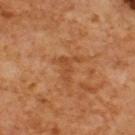The lesion was photographed on a routine skin check and not biopsied; there is no pathology result. Measured at roughly 3.5 mm in maximum diameter. A male patient, aged approximately 60. The lesion is on the upper back. Cropped from a total-body skin-imaging series; the visible field is about 15 mm. An algorithmic analysis of the crop reported an outline eccentricity of about 0.7 (0 = round, 1 = elongated) and a shape-asymmetry score of about 0.6 (0 = symmetric). The software also gave a lesion color around L≈43 a*≈23 b*≈36 in CIELAB, a lesion–skin lightness drop of about 6, and a lesion-to-skin contrast of about 5 (normalized; higher = more distinct). The analysis additionally found a border-irregularity index near 8/10, internal color variation of about 1.5 on a 0–10 scale, and radial color variation of about 0.5. The software also gave lesion-presence confidence of about 95/100.